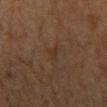Q: Is there a histopathology result?
A: catalogued during a skin exam; not biopsied
Q: What is the imaging modality?
A: ~15 mm tile from a whole-body skin photo
Q: What are the patient's age and sex?
A: male, aged 43 to 47
Q: What is the anatomic site?
A: the head or neck